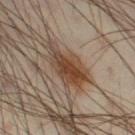Captured during whole-body skin photography for melanoma surveillance; the lesion was not biopsied.
The tile uses cross-polarized illumination.
About 6 mm across.
The patient is a male approximately 40 years of age.
A close-up tile cropped from a whole-body skin photograph, about 15 mm across.
The lesion-visualizer software estimated a footprint of about 13 mm², a shape eccentricity near 0.8, and a symmetry-axis asymmetry near 0.35. And it measured border irregularity of about 4.5 on a 0–10 scale, internal color variation of about 6 on a 0–10 scale, and a peripheral color-asymmetry measure near 2.
From the right thigh.